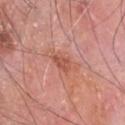follow-up=total-body-photography surveillance lesion; no biopsy | patient=male, aged around 80 | imaging modality=total-body-photography crop, ~15 mm field of view | diameter=~2.5 mm (longest diameter) | tile lighting=white-light illumination | body site=the head or neck.